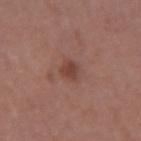notes: total-body-photography surveillance lesion; no biopsy
acquisition: 15 mm crop, total-body photography
diameter: ≈2.5 mm
body site: the left forearm
illumination: white-light
patient: female, in their 50s